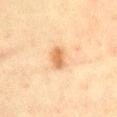Clinical impression: No biopsy was performed on this lesion — it was imaged during a full skin examination and was not determined to be concerning. Acquisition and patient details: About 3 mm across. An algorithmic analysis of the crop reported an area of roughly 5 mm² and two-axis asymmetry of about 0.2. The analysis additionally found a border-irregularity index near 2/10, a within-lesion color-variation index near 2.5/10, and radial color variation of about 1. The software also gave an automated nevus-likeness rating near 80 out of 100 and a lesion-detection confidence of about 100/100. Imaged with cross-polarized lighting. The lesion is located on the abdomen. A region of skin cropped from a whole-body photographic capture, roughly 15 mm wide. A female subject aged 53 to 57.